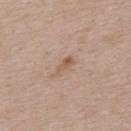| key | value |
|---|---|
| notes | imaged on a skin check; not biopsied |
| site | the upper back |
| image | total-body-photography crop, ~15 mm field of view |
| patient | male, aged 73–77 |
| lesion diameter | ≈2.5 mm |
| lighting | white-light |